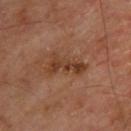notes: total-body-photography surveillance lesion; no biopsy | body site: the back | image source: ~15 mm crop, total-body skin-cancer survey | patient: male, aged approximately 65 | diameter: ≈4.5 mm | tile lighting: cross-polarized illumination.